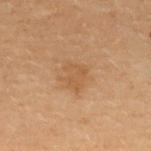<record>
<lesion_size>
  <long_diameter_mm_approx>3.0</long_diameter_mm_approx>
</lesion_size>
<patient>
  <sex>female</sex>
  <age_approx>30</age_approx>
</patient>
<image>
  <source>total-body photography crop</source>
  <field_of_view_mm>15</field_of_view_mm>
</image>
<site>upper back</site>
<lighting>cross-polarized</lighting>
</record>